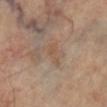| key | value |
|---|---|
| notes | total-body-photography surveillance lesion; no biopsy |
| patient | female, aged 58–62 |
| automated lesion analysis | a lesion–skin lightness drop of about 5 and a normalized border contrast of about 5 |
| lighting | cross-polarized |
| anatomic site | the right leg |
| acquisition | 15 mm crop, total-body photography |
| lesion size | about 3 mm |